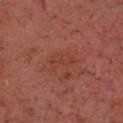follow-up = total-body-photography surveillance lesion; no biopsy
image source = ~15 mm crop, total-body skin-cancer survey
automated lesion analysis = a lesion color around L≈41 a*≈27 b*≈29 in CIELAB, a lesion–skin lightness drop of about 4, and a normalized lesion–skin contrast near 4.5; a border-irregularity index near 4/10, a within-lesion color-variation index near 2/10, and radial color variation of about 0.5
size = ≈3.5 mm
subject = male, roughly 55 years of age
anatomic site = the head or neck
lighting = cross-polarized